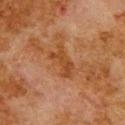workup: imaged on a skin check; not biopsied | lighting: cross-polarized illumination | image-analysis metrics: an area of roughly 6.5 mm² and a shape eccentricity near 0.85; an average lesion color of about L≈35 a*≈20 b*≈32 (CIELAB), about 6 CIELAB-L* units darker than the surrounding skin, and a lesion-to-skin contrast of about 6.5 (normalized; higher = more distinct); a border-irregularity index near 5/10, a within-lesion color-variation index near 2/10, and radial color variation of about 1; a nevus-likeness score of about 0/100 and lesion-presence confidence of about 100/100 | size: about 4 mm | subject: male, aged 78 to 82 | body site: the back | image source: 15 mm crop, total-body photography.